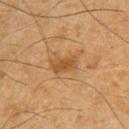patient = male, in their mid- to late 60s | body site = the arm | image source = ~15 mm tile from a whole-body skin photo.